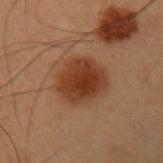{"biopsy_status": "not biopsied; imaged during a skin examination", "site": "arm", "patient": {"sex": "male", "age_approx": 55}, "image": {"source": "total-body photography crop", "field_of_view_mm": 15}, "lesion_size": {"long_diameter_mm_approx": 5.5}, "lighting": "cross-polarized"}